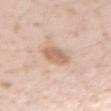<tbp_lesion>
  <biopsy_status>not biopsied; imaged during a skin examination</biopsy_status>
  <image>
    <source>total-body photography crop</source>
    <field_of_view_mm>15</field_of_view_mm>
  </image>
  <site>left forearm</site>
  <patient>
    <sex>female</sex>
    <age_approx>20</age_approx>
  </patient>
</tbp_lesion>